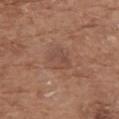Assessment:
Recorded during total-body skin imaging; not selected for excision or biopsy.
Acquisition and patient details:
Captured under white-light illumination. A female subject in their mid- to late 70s. From the upper back. Automated image analysis of the tile measured an eccentricity of roughly 0.55. About 3.5 mm across. Cropped from a whole-body photographic skin survey; the tile spans about 15 mm.